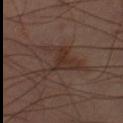follow-up=no biopsy performed (imaged during a skin exam) | patient=male, aged 58 to 62 | image source=total-body-photography crop, ~15 mm field of view | TBP lesion metrics=an area of roughly 12 mm² and an eccentricity of roughly 0.75; a lesion–skin lightness drop of about 6 | location=the left thigh | diameter=about 7 mm.